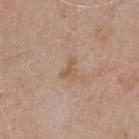Impression: Part of a total-body skin-imaging series; this lesion was reviewed on a skin check and was not flagged for biopsy. Context: The patient is a male aged 73–77. From the front of the torso. An algorithmic analysis of the crop reported a lesion area of about 3 mm², an eccentricity of roughly 0.8, and a symmetry-axis asymmetry near 0.5. The software also gave a border-irregularity rating of about 5/10 and radial color variation of about 0. It also reported a nevus-likeness score of about 0/100. Imaged with white-light lighting. A roughly 15 mm field-of-view crop from a total-body skin photograph.